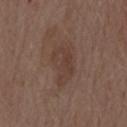Part of a total-body skin-imaging series; this lesion was reviewed on a skin check and was not flagged for biopsy.
On the mid back.
The subject is a male aged around 70.
A close-up tile cropped from a whole-body skin photograph, about 15 mm across.